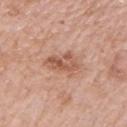| field | value |
|---|---|
| workup | imaged on a skin check; not biopsied |
| tile lighting | white-light illumination |
| size | ≈4 mm |
| image | 15 mm crop, total-body photography |
| anatomic site | the left upper arm |
| patient | female, aged 68–72 |